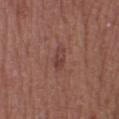No biopsy was performed on this lesion — it was imaged during a full skin examination and was not determined to be concerning. About 2.5 mm across. A female subject about 40 years old. The lesion is located on the leg. A 15 mm crop from a total-body photograph taken for skin-cancer surveillance.